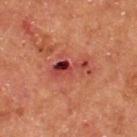{"biopsy_status": "not biopsied; imaged during a skin examination", "site": "upper back", "patient": {"sex": "male", "age_approx": 55}, "lighting": "cross-polarized", "image": {"source": "total-body photography crop", "field_of_view_mm": 15}, "automated_metrics": {"area_mm2_approx": 7.5, "eccentricity": 0.9, "shape_asymmetry": 0.55, "vs_skin_darker_L": 11.0, "border_irregularity_0_10": 7.5, "color_variation_0_10": 9.5, "peripheral_color_asymmetry": 4.0}}